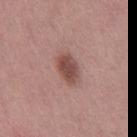The lesion was tiled from a total-body skin photograph and was not biopsied.
Imaged with white-light lighting.
A roughly 15 mm field-of-view crop from a total-body skin photograph.
The lesion is located on the right thigh.
A female patient about 50 years old.